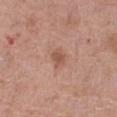follow-up = total-body-photography surveillance lesion; no biopsy | image = ~15 mm crop, total-body skin-cancer survey | tile lighting = white-light illumination | body site = the right thigh | patient = female, in their mid- to late 60s | lesion diameter = ≈2.5 mm.